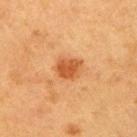Q: Was this lesion biopsied?
A: no biopsy performed (imaged during a skin exam)
Q: Who is the patient?
A: female, roughly 40 years of age
Q: What kind of image is this?
A: 15 mm crop, total-body photography
Q: What is the anatomic site?
A: the right upper arm